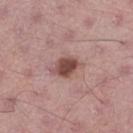{
  "biopsy_status": "not biopsied; imaged during a skin examination",
  "image": {
    "source": "total-body photography crop",
    "field_of_view_mm": 15
  },
  "lighting": "white-light",
  "patient": {
    "sex": "male",
    "age_approx": 65
  },
  "site": "right thigh"
}